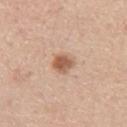Assessment:
Part of a total-body skin-imaging series; this lesion was reviewed on a skin check and was not flagged for biopsy.
Context:
About 2.5 mm across. Located on the right upper arm. This image is a 15 mm lesion crop taken from a total-body photograph. The subject is a male aged around 60. Automated image analysis of the tile measured an area of roughly 5 mm² and a symmetry-axis asymmetry near 0.2. And it measured a lesion color around L≈58 a*≈21 b*≈31 in CIELAB, about 13 CIELAB-L* units darker than the surrounding skin, and a lesion-to-skin contrast of about 9 (normalized; higher = more distinct). The software also gave a border-irregularity rating of about 1.5/10 and a color-variation rating of about 3.5/10. It also reported an automated nevus-likeness rating near 95 out of 100.